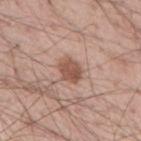notes: catalogued during a skin exam; not biopsied
subject: male, in their mid- to late 50s
body site: the back
tile lighting: white-light
image source: total-body-photography crop, ~15 mm field of view
automated lesion analysis: a footprint of about 6.5 mm², an outline eccentricity of about 0.65 (0 = round, 1 = elongated), and a symmetry-axis asymmetry near 0.2; a border-irregularity rating of about 2/10 and a within-lesion color-variation index near 3/10; a classifier nevus-likeness of about 95/100 and a detector confidence of about 100 out of 100 that the crop contains a lesion